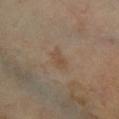The lesion was photographed on a routine skin check and not biopsied; there is no pathology result. This image is a 15 mm lesion crop taken from a total-body photograph. This is a cross-polarized tile. A male patient, aged around 65. Approximately 3 mm at its widest. On the left lower leg.